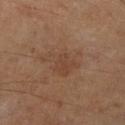notes: no biopsy performed (imaged during a skin exam)
illumination: cross-polarized
image-analysis metrics: a footprint of about 11 mm², an outline eccentricity of about 0.75 (0 = round, 1 = elongated), and two-axis asymmetry of about 0.35; about 5 CIELAB-L* units darker than the surrounding skin and a lesion-to-skin contrast of about 4.5 (normalized; higher = more distinct)
patient: male, in their mid- to late 60s
imaging modality: total-body-photography crop, ~15 mm field of view
lesion diameter: ≈5 mm
location: the right lower leg